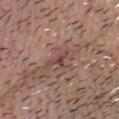Image and clinical context: Longest diameter approximately 3 mm. A male subject in their mid- to late 50s. On the head or neck. A 15 mm close-up tile from a total-body photography series done for melanoma screening. The total-body-photography lesion software estimated a lesion area of about 3 mm², an outline eccentricity of about 0.9 (0 = round, 1 = elongated), and a shape-asymmetry score of about 0.5 (0 = symmetric). Captured under white-light illumination.